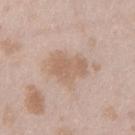A female patient, in their mid- to late 20s. The lesion is on the left thigh. A lesion tile, about 15 mm wide, cut from a 3D total-body photograph. About 5 mm across.